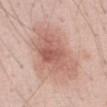Clinical impression: The lesion was tiled from a total-body skin photograph and was not biopsied. Acquisition and patient details: This image is a 15 mm lesion crop taken from a total-body photograph. About 8 mm across. The lesion is on the front of the torso. Imaged with white-light lighting. A male subject, aged 53–57. The total-body-photography lesion software estimated an eccentricity of roughly 0.75 and a shape-asymmetry score of about 0.3 (0 = symmetric). And it measured an average lesion color of about L≈61 a*≈22 b*≈26 (CIELAB), a lesion–skin lightness drop of about 10, and a normalized lesion–skin contrast near 6.5. The analysis additionally found a classifier nevus-likeness of about 35/100 and lesion-presence confidence of about 100/100.